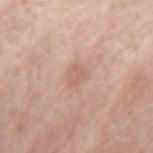Imaged during a routine full-body skin examination; the lesion was not biopsied and no histopathology is available. The tile uses white-light illumination. A female patient, approximately 85 years of age or older. This image is a 15 mm lesion crop taken from a total-body photograph. The lesion is on the right upper arm. Longest diameter approximately 3 mm.